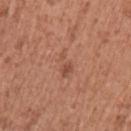Image and clinical context: A region of skin cropped from a whole-body photographic capture, roughly 15 mm wide. Measured at roughly 3.5 mm in maximum diameter. A female patient, aged 48–52. The lesion-visualizer software estimated an area of roughly 4 mm², an eccentricity of roughly 0.9, and a symmetry-axis asymmetry near 0.4. And it measured a mean CIELAB color near L≈51 a*≈24 b*≈31, a lesion–skin lightness drop of about 7, and a lesion-to-skin contrast of about 5 (normalized; higher = more distinct). Imaged with white-light lighting. The lesion is located on the left upper arm.